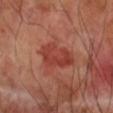Acquisition and patient details: Automated tile analysis of the lesion measured an average lesion color of about L≈41 a*≈31 b*≈29 (CIELAB), about 8 CIELAB-L* units darker than the surrounding skin, and a normalized lesion–skin contrast near 7. It also reported a border-irregularity rating of about 3/10, a within-lesion color-variation index near 3.5/10, and a peripheral color-asymmetry measure near 1.5. A male patient, aged 68 to 72. Longest diameter approximately 4.5 mm. Imaged with cross-polarized lighting. Cropped from a total-body skin-imaging series; the visible field is about 15 mm. The lesion is on the right forearm.